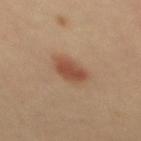biopsy status=no biopsy performed (imaged during a skin exam) | TBP lesion metrics=border irregularity of about 2 on a 0–10 scale and internal color variation of about 2.5 on a 0–10 scale; an automated nevus-likeness rating near 100 out of 100 and lesion-presence confidence of about 100/100 | lighting=cross-polarized | lesion size=~3.5 mm (longest diameter) | patient=female, aged around 50 | acquisition=total-body-photography crop, ~15 mm field of view.